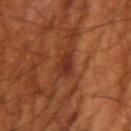| feature | finding |
|---|---|
| follow-up | catalogued during a skin exam; not biopsied |
| subject | male, roughly 55 years of age |
| size | ≈3.5 mm |
| body site | the right upper arm |
| acquisition | total-body-photography crop, ~15 mm field of view |